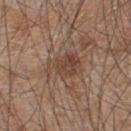This lesion was catalogued during total-body skin photography and was not selected for biopsy. On the upper back. The total-body-photography lesion software estimated a footprint of about 10 mm², an eccentricity of roughly 0.65, and a shape-asymmetry score of about 0.35 (0 = symmetric). The patient is a male aged 43 to 47. A 15 mm crop from a total-body photograph taken for skin-cancer surveillance. The lesion's longest dimension is about 4.5 mm.